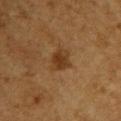Captured during whole-body skin photography for melanoma surveillance; the lesion was not biopsied. Imaged with cross-polarized lighting. The lesion's longest dimension is about 2.5 mm. A 15 mm close-up tile from a total-body photography series done for melanoma screening. The lesion-visualizer software estimated a lesion area of about 5 mm² and a shape eccentricity near 0.4. The analysis additionally found an average lesion color of about L≈32 a*≈18 b*≈31 (CIELAB), about 8 CIELAB-L* units darker than the surrounding skin, and a lesion-to-skin contrast of about 8 (normalized; higher = more distinct). Located on the chest. The patient is a male in their mid-50s.